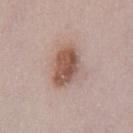The lesion was tiled from a total-body skin photograph and was not biopsied. Cropped from a whole-body photographic skin survey; the tile spans about 15 mm. A female patient, aged approximately 30. Measured at roughly 5 mm in maximum diameter. On the chest. The tile uses white-light illumination.